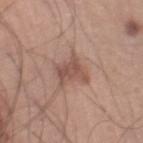Notes:
• workup — total-body-photography surveillance lesion; no biopsy
• size — ~3.5 mm (longest diameter)
• tile lighting — white-light
• image — total-body-photography crop, ~15 mm field of view
• body site — the right lower leg
• image-analysis metrics — peripheral color asymmetry of about 0.5; a nevus-likeness score of about 10/100 and lesion-presence confidence of about 100/100
• subject — male, approximately 45 years of age